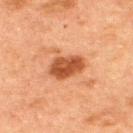Notes:
* follow-up · no biopsy performed (imaged during a skin exam)
* anatomic site · the upper back
* lighting · cross-polarized
* automated metrics · a lesion area of about 9 mm², an outline eccentricity of about 0.75 (0 = round, 1 = elongated), and a symmetry-axis asymmetry near 0.2; a border-irregularity rating of about 2/10 and a color-variation rating of about 3/10; a detector confidence of about 100 out of 100 that the crop contains a lesion
* imaging modality · ~15 mm crop, total-body skin-cancer survey
* subject · male, about 50 years old
* lesion diameter · ~4 mm (longest diameter)